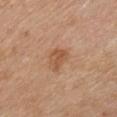The lesion was tiled from a total-body skin photograph and was not biopsied.
Located on the chest.
Measured at roughly 2.5 mm in maximum diameter.
A 15 mm close-up extracted from a 3D total-body photography capture.
An algorithmic analysis of the crop reported a shape eccentricity near 0.5. And it measured an average lesion color of about L≈54 a*≈22 b*≈34 (CIELAB), a lesion–skin lightness drop of about 8, and a lesion-to-skin contrast of about 6.5 (normalized; higher = more distinct).
A male subject in their mid-50s.
Captured under white-light illumination.